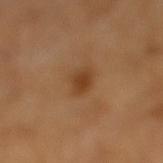Assessment:
This lesion was catalogued during total-body skin photography and was not selected for biopsy.
Context:
From the right lower leg. The subject is a female aged 53 to 57. Cropped from a total-body skin-imaging series; the visible field is about 15 mm. Captured under cross-polarized illumination. Automated tile analysis of the lesion measured a lesion area of about 4.5 mm², an outline eccentricity of about 0.65 (0 = round, 1 = elongated), and two-axis asymmetry of about 0.2. The analysis additionally found a border-irregularity rating of about 2/10 and internal color variation of about 3 on a 0–10 scale. And it measured a classifier nevus-likeness of about 90/100 and a lesion-detection confidence of about 100/100.